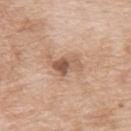biopsy status = no biopsy performed (imaged during a skin exam) | subject = male, in their 70s | imaging modality = ~15 mm tile from a whole-body skin photo | lesion size = about 4 mm | tile lighting = white-light | site = the upper back.